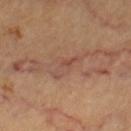{"biopsy_status": "not biopsied; imaged during a skin examination", "lighting": "cross-polarized", "image": {"source": "total-body photography crop", "field_of_view_mm": 15}, "lesion_size": {"long_diameter_mm_approx": 4.5}, "automated_metrics": {"border_irregularity_0_10": 5.5, "nevus_likeness_0_100": 0, "lesion_detection_confidence_0_100": 70}, "site": "left thigh", "patient": {"sex": "female", "age_approx": 60}}